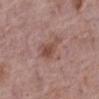| field | value |
|---|---|
| notes | imaged on a skin check; not biopsied |
| imaging modality | total-body-photography crop, ~15 mm field of view |
| patient | female, about 65 years old |
| body site | the left lower leg |
| size | ≈4 mm |
| lighting | white-light |